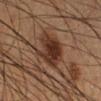notes: catalogued during a skin exam; not biopsied
location: the right lower leg
acquisition: total-body-photography crop, ~15 mm field of view
illumination: cross-polarized illumination
subject: male, aged 58–62